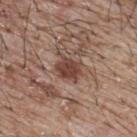{
  "biopsy_status": "not biopsied; imaged during a skin examination",
  "image": {
    "source": "total-body photography crop",
    "field_of_view_mm": 15
  },
  "lighting": "white-light",
  "site": "upper back",
  "lesion_size": {
    "long_diameter_mm_approx": 3.5
  },
  "patient": {
    "sex": "male",
    "age_approx": 65
  }
}